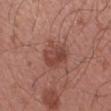Notes:
* notes — no biopsy performed (imaged during a skin exam)
* subject — male, approximately 35 years of age
* location — the left forearm
* lighting — white-light illumination
* acquisition — ~15 mm crop, total-body skin-cancer survey
* lesion size — ~4 mm (longest diameter)
* TBP lesion metrics — a lesion area of about 10 mm² and a shape eccentricity near 0.6